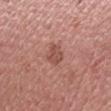Automated image analysis of the tile measured a border-irregularity rating of about 3.5/10, internal color variation of about 2 on a 0–10 scale, and radial color variation of about 0.5. A male patient, about 30 years old. The tile uses white-light illumination. The lesion's longest dimension is about 2.5 mm. This image is a 15 mm lesion crop taken from a total-body photograph.